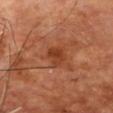Impression:
Part of a total-body skin-imaging series; this lesion was reviewed on a skin check and was not flagged for biopsy.
Acquisition and patient details:
The lesion is on the chest. This is a cross-polarized tile. The lesion's longest dimension is about 3 mm. This image is a 15 mm lesion crop taken from a total-body photograph. A male patient, aged 68 to 72.